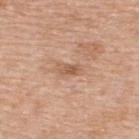Findings:
* notes: no biopsy performed (imaged during a skin exam)
* body site: the upper back
* automated metrics: an eccentricity of roughly 0.85 and two-axis asymmetry of about 0.3
* image source: ~15 mm crop, total-body skin-cancer survey
* illumination: white-light
* patient: female, aged approximately 55
* lesion size: ~2.5 mm (longest diameter)